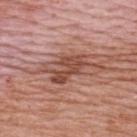Notes:
- follow-up · no biopsy performed (imaged during a skin exam)